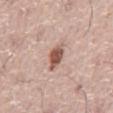Notes:
– follow-up — total-body-photography surveillance lesion; no biopsy
– imaging modality — total-body-photography crop, ~15 mm field of view
– patient — male, about 75 years old
– site — the abdomen